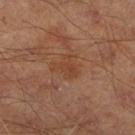Impression: This lesion was catalogued during total-body skin photography and was not selected for biopsy.